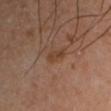No biopsy was performed on this lesion — it was imaged during a full skin examination and was not determined to be concerning. The lesion is located on the left upper arm. The patient is a male in their 40s. A 15 mm close-up extracted from a 3D total-body photography capture.